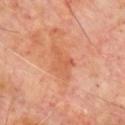Clinical summary: A male subject, aged around 65. The lesion is located on the chest. A close-up tile cropped from a whole-body skin photograph, about 15 mm across. The tile uses cross-polarized illumination. The recorded lesion diameter is about 4 mm. Automated tile analysis of the lesion measured a lesion area of about 7 mm² and an eccentricity of roughly 0.75. It also reported a border-irregularity index near 5.5/10, internal color variation of about 3.5 on a 0–10 scale, and radial color variation of about 1.5. And it measured a detector confidence of about 100 out of 100 that the crop contains a lesion.